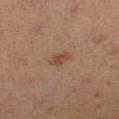  biopsy_status: not biopsied; imaged during a skin examination
  site: left lower leg
  image:
    source: total-body photography crop
    field_of_view_mm: 15
  patient:
    sex: female
    age_approx: 35